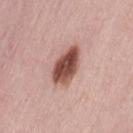Q: Was this lesion biopsied?
A: catalogued during a skin exam; not biopsied